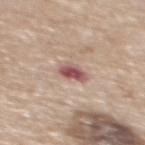Case summary:
* subject · female, aged 63 to 67
* location · the upper back
* tile lighting · white-light
* acquisition · ~15 mm tile from a whole-body skin photo